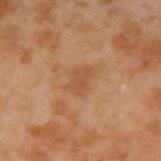Q: What is the lesion's diameter?
A: ≈3.5 mm
Q: What is the imaging modality?
A: total-body-photography crop, ~15 mm field of view
Q: What did automated image analysis measure?
A: a color-variation rating of about 1/10 and a peripheral color-asymmetry measure near 0.5; an automated nevus-likeness rating near 0 out of 100 and a lesion-detection confidence of about 100/100
Q: What are the patient's age and sex?
A: male, about 45 years old
Q: Where on the body is the lesion?
A: the right forearm